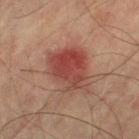The lesion was tiled from a total-body skin photograph and was not biopsied.
On the right thigh.
Approximately 5.5 mm at its widest.
Imaged with cross-polarized lighting.
The patient is a male in their mid-70s.
A 15 mm crop from a total-body photograph taken for skin-cancer surveillance.